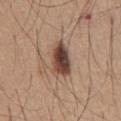{"biopsy_status": "not biopsied; imaged during a skin examination", "site": "abdomen", "patient": {"sex": "male", "age_approx": 65}, "automated_metrics": {"area_mm2_approx": 10.0, "eccentricity": 0.85, "shape_asymmetry": 0.2, "cielab_L": 45, "cielab_a": 17, "cielab_b": 25, "vs_skin_darker_L": 17.0, "vs_skin_contrast_norm": 12.0, "border_irregularity_0_10": 2.5, "color_variation_0_10": 7.5, "peripheral_color_asymmetry": 2.0, "nevus_likeness_0_100": 95, "lesion_detection_confidence_0_100": 100}, "lesion_size": {"long_diameter_mm_approx": 5.0}, "image": {"source": "total-body photography crop", "field_of_view_mm": 15}}